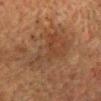{
  "biopsy_status": "not biopsied; imaged during a skin examination",
  "automated_metrics": {
    "cielab_L": 32,
    "cielab_a": 16,
    "cielab_b": 25,
    "vs_skin_darker_L": 5.0,
    "vs_skin_contrast_norm": 5.0,
    "nevus_likeness_0_100": 5,
    "lesion_detection_confidence_0_100": 100
  },
  "lesion_size": {
    "long_diameter_mm_approx": 5.5
  },
  "patient": {
    "sex": "male",
    "age_approx": 60
  },
  "lighting": "cross-polarized",
  "site": "head or neck",
  "image": {
    "source": "total-body photography crop",
    "field_of_view_mm": 15
  }
}